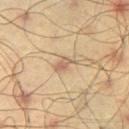Case summary:
* follow-up: catalogued during a skin exam; not biopsied
* site: the left thigh
* image: 15 mm crop, total-body photography
* patient: male, in their mid- to late 40s
* lesion size: ≈3 mm